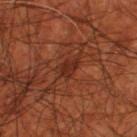This lesion was catalogued during total-body skin photography and was not selected for biopsy. The lesion-visualizer software estimated a lesion area of about 4 mm², an outline eccentricity of about 0.75 (0 = round, 1 = elongated), and two-axis asymmetry of about 0.3. The software also gave a lesion color around L≈28 a*≈25 b*≈29 in CIELAB, a lesion–skin lightness drop of about 7, and a normalized border contrast of about 7.5. The analysis additionally found border irregularity of about 3 on a 0–10 scale and a within-lesion color-variation index near 1.5/10. The analysis additionally found an automated nevus-likeness rating near 0 out of 100 and a detector confidence of about 85 out of 100 that the crop contains a lesion. A male subject, aged around 70. The recorded lesion diameter is about 2.5 mm. Captured under cross-polarized illumination. The lesion is on the right thigh. A close-up tile cropped from a whole-body skin photograph, about 15 mm across.